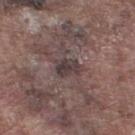  biopsy_status: not biopsied; imaged during a skin examination
  image:
    source: total-body photography crop
    field_of_view_mm: 15
  patient:
    sex: male
    age_approx: 75
  automated_metrics:
    cielab_L: 37
    cielab_a: 13
    cielab_b: 14
    vs_skin_darker_L: 9.0
    vs_skin_contrast_norm: 8.5
    color_variation_0_10: 3.5
    peripheral_color_asymmetry: 1.0
    nevus_likeness_0_100: 0
  site: right lower leg